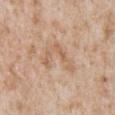follow-up=imaged on a skin check; not biopsied
imaging modality=total-body-photography crop, ~15 mm field of view
diameter=≈4.5 mm
tile lighting=white-light illumination
body site=the chest
subject=male, about 65 years old